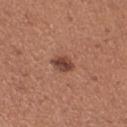Part of a total-body skin-imaging series; this lesion was reviewed on a skin check and was not flagged for biopsy. Approximately 3 mm at its widest. A roughly 15 mm field-of-view crop from a total-body skin photograph. The tile uses white-light illumination. Located on the left thigh. A female patient approximately 35 years of age.